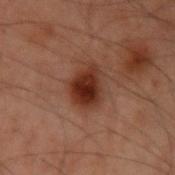<record>
  <biopsy_status>not biopsied; imaged during a skin examination</biopsy_status>
  <image>
    <source>total-body photography crop</source>
    <field_of_view_mm>15</field_of_view_mm>
  </image>
  <automated_metrics>
    <cielab_L>24</cielab_L>
    <cielab_a>19</cielab_a>
    <cielab_b>22</cielab_b>
    <vs_skin_contrast_norm>11.0</vs_skin_contrast_norm>
  </automated_metrics>
  <patient>
    <sex>male</sex>
    <age_approx>60</age_approx>
  </patient>
  <site>arm</site>
</record>